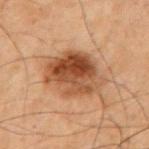| feature | finding |
|---|---|
| notes | imaged on a skin check; not biopsied |
| subject | male, aged 68–72 |
| site | the front of the torso |
| acquisition | ~15 mm crop, total-body skin-cancer survey |